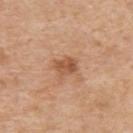No biopsy was performed on this lesion — it was imaged during a full skin examination and was not determined to be concerning. From the back. The total-body-photography lesion software estimated a footprint of about 4.5 mm² and a shape-asymmetry score of about 0.35 (0 = symmetric). The tile uses white-light illumination. The patient is a male aged 58 to 62. Cropped from a whole-body photographic skin survey; the tile spans about 15 mm. About 3 mm across.